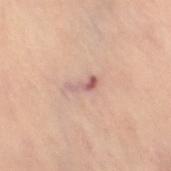notes: no biopsy performed (imaged during a skin exam); patient: female, about 60 years old; lesion size: ~3 mm (longest diameter); site: the left leg; image: 15 mm crop, total-body photography; tile lighting: cross-polarized.